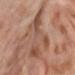Notes:
- notes — total-body-photography surveillance lesion; no biopsy
- lesion diameter — about 7.5 mm
- location — the right lower leg
- patient — female, about 55 years old
- TBP lesion metrics — a lesion area of about 22 mm², a shape eccentricity near 0.85, and a symmetry-axis asymmetry near 0.55; a mean CIELAB color near L≈52 a*≈21 b*≈28, about 9 CIELAB-L* units darker than the surrounding skin, and a lesion-to-skin contrast of about 6 (normalized; higher = more distinct); a border-irregularity index near 9/10, a color-variation rating of about 6/10, and radial color variation of about 1.5
- imaging modality — total-body-photography crop, ~15 mm field of view
- lighting — cross-polarized illumination